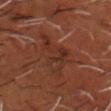<tbp_lesion>
<biopsy_status>not biopsied; imaged during a skin examination</biopsy_status>
<patient>
  <sex>male</sex>
  <age_approx>50</age_approx>
</patient>
<image>
  <source>total-body photography crop</source>
  <field_of_view_mm>15</field_of_view_mm>
</image>
<lighting>cross-polarized</lighting>
<site>head or neck</site>
</tbp_lesion>